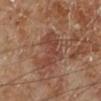{
  "biopsy_status": "not biopsied; imaged during a skin examination",
  "site": "leg",
  "patient": {
    "sex": "male",
    "age_approx": 70
  },
  "image": {
    "source": "total-body photography crop",
    "field_of_view_mm": 15
  }
}